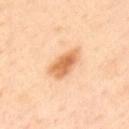Captured during whole-body skin photography for melanoma surveillance; the lesion was not biopsied. A male patient roughly 45 years of age. Approximately 5 mm at its widest. The lesion is located on the upper back. An algorithmic analysis of the crop reported a mean CIELAB color near L≈68 a*≈23 b*≈41, about 13 CIELAB-L* units darker than the surrounding skin, and a normalized lesion–skin contrast near 8.5. It also reported a border-irregularity rating of about 2.5/10, a within-lesion color-variation index near 4/10, and peripheral color asymmetry of about 1. And it measured an automated nevus-likeness rating near 95 out of 100 and a lesion-detection confidence of about 100/100. The tile uses cross-polarized illumination. A close-up tile cropped from a whole-body skin photograph, about 15 mm across.